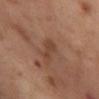On the left thigh. About 3 mm across. The subject is a female aged around 55. A close-up tile cropped from a whole-body skin photograph, about 15 mm across. This is a cross-polarized tile.